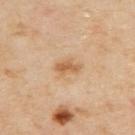{
  "biopsy_status": "not biopsied; imaged during a skin examination",
  "site": "back",
  "lighting": "cross-polarized",
  "image": {
    "source": "total-body photography crop",
    "field_of_view_mm": 15
  },
  "lesion_size": {
    "long_diameter_mm_approx": 3.0
  },
  "patient": {
    "sex": "female",
    "age_approx": 60
  }
}